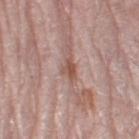Notes:
• follow-up · total-body-photography surveillance lesion; no biopsy
• illumination · white-light illumination
• acquisition · ~15 mm crop, total-body skin-cancer survey
• patient · female, roughly 65 years of age
• site · the left leg